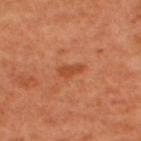Part of a total-body skin-imaging series; this lesion was reviewed on a skin check and was not flagged for biopsy. Approximately 2.5 mm at its widest. This image is a 15 mm lesion crop taken from a total-body photograph. A male subject about 50 years old. The lesion is located on the back.